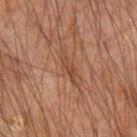  biopsy_status: not biopsied; imaged during a skin examination
  lesion_size:
    long_diameter_mm_approx: 3.5
  image:
    source: total-body photography crop
    field_of_view_mm: 15
  site: left upper arm
  lighting: cross-polarized
  patient:
    sex: male
    age_approx: 65